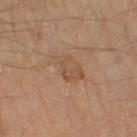<lesion>
<biopsy_status>not biopsied; imaged during a skin examination</biopsy_status>
<lighting>cross-polarized</lighting>
<image>
  <source>total-body photography crop</source>
  <field_of_view_mm>15</field_of_view_mm>
</image>
<site>leg</site>
<lesion_size>
  <long_diameter_mm_approx>4.0</long_diameter_mm_approx>
</lesion_size>
<patient>
  <age_approx>55</age_approx>
</patient>
</lesion>